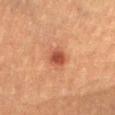Findings:
– notes: total-body-photography surveillance lesion; no biopsy
– subject: female, aged around 70
– location: the lower back
– image source: ~15 mm tile from a whole-body skin photo